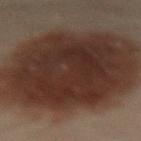The lesion was tiled from a total-body skin photograph and was not biopsied. This image is a 15 mm lesion crop taken from a total-body photograph. A male patient approximately 50 years of age. Located on the lower back.